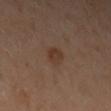The lesion was tiled from a total-body skin photograph and was not biopsied. A roughly 15 mm field-of-view crop from a total-body skin photograph. The subject is a female aged 38–42. Measured at roughly 2.5 mm in maximum diameter. Automated image analysis of the tile measured a lesion area of about 4 mm², an outline eccentricity of about 0.7 (0 = round, 1 = elongated), and a shape-asymmetry score of about 0.2 (0 = symmetric). And it measured a mean CIELAB color near L≈36 a*≈17 b*≈27, a lesion–skin lightness drop of about 6, and a normalized border contrast of about 7. The software also gave a border-irregularity rating of about 1.5/10. And it measured a classifier nevus-likeness of about 80/100. This is a cross-polarized tile. Located on the left upper arm.